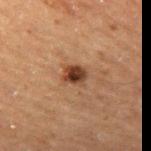Located on the leg. Longest diameter approximately 2.5 mm. A male subject about 70 years old. The total-body-photography lesion software estimated a footprint of about 4.5 mm², a shape eccentricity near 0.6, and a symmetry-axis asymmetry near 0.15. And it measured an average lesion color of about L≈30 a*≈18 b*≈25 (CIELAB), roughly 13 lightness units darker than nearby skin, and a lesion-to-skin contrast of about 12 (normalized; higher = more distinct). This image is a 15 mm lesion crop taken from a total-body photograph.